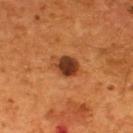| field | value |
|---|---|
| subject | male, aged around 55 |
| image | ~15 mm tile from a whole-body skin photo |
| lesion size | about 4 mm |
| anatomic site | the back |
| automated metrics | an area of roughly 8 mm² and an eccentricity of roughly 0.75; a classifier nevus-likeness of about 80/100 and a detector confidence of about 100 out of 100 that the crop contains a lesion |
| tile lighting | cross-polarized illumination |